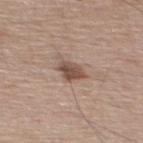biopsy_status: not biopsied; imaged during a skin examination
patient:
  sex: male
  age_approx: 55
image:
  source: total-body photography crop
  field_of_view_mm: 15
site: mid back
lighting: white-light